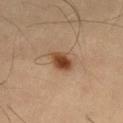Background:
On the leg. Approximately 3 mm at its widest. The lesion-visualizer software estimated a lesion area of about 5.5 mm², a shape eccentricity near 0.55, and a shape-asymmetry score of about 0.15 (0 = symmetric). The analysis additionally found a border-irregularity index near 1.5/10, internal color variation of about 4.5 on a 0–10 scale, and peripheral color asymmetry of about 1.5. It also reported an automated nevus-likeness rating near 100 out of 100 and a detector confidence of about 100 out of 100 that the crop contains a lesion. A male subject, aged around 55. Captured under cross-polarized illumination. A close-up tile cropped from a whole-body skin photograph, about 15 mm across.